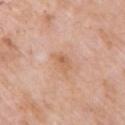Clinical impression: Imaged during a routine full-body skin examination; the lesion was not biopsied and no histopathology is available. Acquisition and patient details: From the right upper arm. This image is a 15 mm lesion crop taken from a total-body photograph. The patient is a female aged approximately 70.